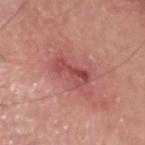Findings:
* biopsy status: total-body-photography surveillance lesion; no biopsy
* subject: male, about 65 years old
* illumination: white-light
* imaging modality: ~15 mm tile from a whole-body skin photo
* lesion diameter: ≈4 mm
* location: the head or neck